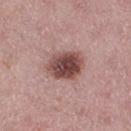Impression:
The lesion was tiled from a total-body skin photograph and was not biopsied.
Background:
The lesion is located on the right thigh. A close-up tile cropped from a whole-body skin photograph, about 15 mm across. Captured under white-light illumination. Measured at roughly 4 mm in maximum diameter. A female patient, about 45 years old.